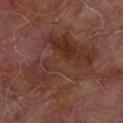Impression: This lesion was catalogued during total-body skin photography and was not selected for biopsy. Acquisition and patient details: The lesion-visualizer software estimated a lesion color around L≈26 a*≈17 b*≈21 in CIELAB, roughly 5 lightness units darker than nearby skin, and a lesion-to-skin contrast of about 6 (normalized; higher = more distinct). The analysis additionally found a border-irregularity rating of about 9/10, a color-variation rating of about 5/10, and peripheral color asymmetry of about 1.5. About 9 mm across. From the right forearm. A 15 mm crop from a total-body photograph taken for skin-cancer surveillance. The patient is a male aged 73–77.